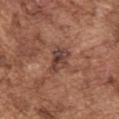<lesion>
  <biopsy_status>not biopsied; imaged during a skin examination</biopsy_status>
  <lesion_size>
    <long_diameter_mm_approx>3.5</long_diameter_mm_approx>
  </lesion_size>
  <automated_metrics>
    <area_mm2_approx>6.5</area_mm2_approx>
    <eccentricity>0.8</eccentricity>
    <shape_asymmetry>0.3</shape_asymmetry>
    <cielab_L>42</cielab_L>
    <cielab_a>21</cielab_a>
    <cielab_b>24</cielab_b>
    <vs_skin_darker_L>10.0</vs_skin_darker_L>
    <vs_skin_contrast_norm>9.0</vs_skin_contrast_norm>
    <border_irregularity_0_10>3.5</border_irregularity_0_10>
    <color_variation_0_10>6.0</color_variation_0_10>
    <lesion_detection_confidence_0_100>95</lesion_detection_confidence_0_100>
  </automated_metrics>
  <patient>
    <sex>male</sex>
    <age_approx>75</age_approx>
  </patient>
  <site>left upper arm</site>
  <lighting>white-light</lighting>
  <image>
    <source>total-body photography crop</source>
    <field_of_view_mm>15</field_of_view_mm>
  </image>
</lesion>